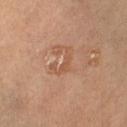Notes:
• follow-up: no biopsy performed (imaged during a skin exam)
• illumination: cross-polarized
• automated lesion analysis: an area of roughly 4 mm², an eccentricity of roughly 0.85, and a symmetry-axis asymmetry near 0.75; a mean CIELAB color near L≈51 a*≈21 b*≈31, roughly 7 lightness units darker than nearby skin, and a lesion-to-skin contrast of about 5.5 (normalized; higher = more distinct); a within-lesion color-variation index near 0/10 and a peripheral color-asymmetry measure near 0
• anatomic site: the left thigh
• imaging modality: 15 mm crop, total-body photography
• subject: female, aged 63–67